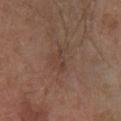This lesion was catalogued during total-body skin photography and was not selected for biopsy. Longest diameter approximately 2.5 mm. A male patient, aged 63 to 67. Captured under white-light illumination. Cropped from a whole-body photographic skin survey; the tile spans about 15 mm. From the right lower leg.